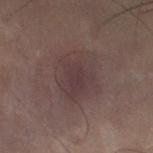Impression:
Recorded during total-body skin imaging; not selected for excision or biopsy.
Acquisition and patient details:
A male patient approximately 50 years of age. A region of skin cropped from a whole-body photographic capture, roughly 15 mm wide. The recorded lesion diameter is about 6 mm. From the left lower leg.